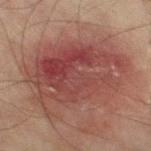Captured under cross-polarized illumination. Measured at roughly 10.5 mm in maximum diameter. A close-up tile cropped from a whole-body skin photograph, about 15 mm across. On the right thigh. A male subject aged approximately 75. The total-body-photography lesion software estimated a footprint of about 60 mm², an eccentricity of roughly 0.7, and two-axis asymmetry of about 0.2. The analysis additionally found a lesion-detection confidence of about 100/100.